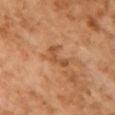Impression:
Imaged during a routine full-body skin examination; the lesion was not biopsied and no histopathology is available.
Image and clinical context:
The total-body-photography lesion software estimated an average lesion color of about L≈44 a*≈21 b*≈33 (CIELAB), a lesion–skin lightness drop of about 8, and a lesion-to-skin contrast of about 6.5 (normalized; higher = more distinct). A 15 mm close-up extracted from a 3D total-body photography capture. About 3.5 mm across. A female subject aged approximately 60. Located on the front of the torso.